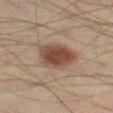workup = catalogued during a skin exam; not biopsied
acquisition = total-body-photography crop, ~15 mm field of view
body site = the left thigh
automated metrics = a footprint of about 14 mm² and two-axis asymmetry of about 0.2; a nevus-likeness score of about 100/100 and lesion-presence confidence of about 100/100
size = ~5 mm (longest diameter)
patient = male, about 40 years old
tile lighting = cross-polarized illumination